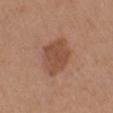Context: The lesion's longest dimension is about 4.5 mm. On the right upper arm. The tile uses white-light illumination. Cropped from a whole-body photographic skin survey; the tile spans about 15 mm. The subject is a female aged 38–42.